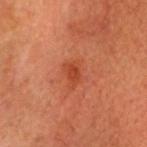This lesion was catalogued during total-body skin photography and was not selected for biopsy.
This is a cross-polarized tile.
A lesion tile, about 15 mm wide, cut from a 3D total-body photograph.
A male patient about 60 years old.
The total-body-photography lesion software estimated a border-irregularity rating of about 2.5/10, a color-variation rating of about 1.5/10, and peripheral color asymmetry of about 0.5. It also reported a nevus-likeness score of about 45/100.
The lesion is located on the head or neck.